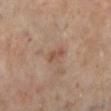follow-up: no biopsy performed (imaged during a skin exam)
lesion size: about 2.5 mm
imaging modality: ~15 mm crop, total-body skin-cancer survey
automated metrics: a footprint of about 2.5 mm², an outline eccentricity of about 0.9 (0 = round, 1 = elongated), and a shape-asymmetry score of about 0.25 (0 = symmetric); a border-irregularity index near 2.5/10, a within-lesion color-variation index near 0/10, and peripheral color asymmetry of about 0; a nevus-likeness score of about 5/100 and lesion-presence confidence of about 100/100
body site: the left lower leg
subject: female, aged approximately 60
illumination: cross-polarized illumination